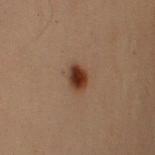Q: Was this lesion biopsied?
A: catalogued during a skin exam; not biopsied
Q: Illumination type?
A: cross-polarized illumination
Q: What did automated image analysis measure?
A: a footprint of about 5 mm² and a shape-asymmetry score of about 0.15 (0 = symmetric); a normalized lesion–skin contrast near 12; a nevus-likeness score of about 100/100
Q: Where on the body is the lesion?
A: the right upper arm
Q: How was this image acquired?
A: ~15 mm crop, total-body skin-cancer survey
Q: Who is the patient?
A: male, aged 53 to 57